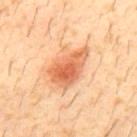Q: Is there a histopathology result?
A: total-body-photography surveillance lesion; no biopsy
Q: How was this image acquired?
A: total-body-photography crop, ~15 mm field of view
Q: What are the patient's age and sex?
A: male, aged around 30
Q: Lesion location?
A: the upper back